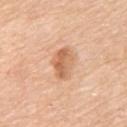automated lesion analysis: an area of roughly 8.5 mm², an outline eccentricity of about 0.75 (0 = round, 1 = elongated), and a shape-asymmetry score of about 0.25 (0 = symmetric); a mean CIELAB color near L≈64 a*≈22 b*≈36 and a normalized border contrast of about 7; border irregularity of about 2.5 on a 0–10 scale and radial color variation of about 2; a classifier nevus-likeness of about 15/100 and a detector confidence of about 100 out of 100 that the crop contains a lesion | lighting: white-light | image: total-body-photography crop, ~15 mm field of view | patient: female, about 65 years old | anatomic site: the upper back.